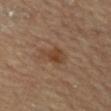{
  "biopsy_status": "not biopsied; imaged during a skin examination",
  "lighting": "cross-polarized",
  "lesion_size": {
    "long_diameter_mm_approx": 4.0
  },
  "site": "mid back",
  "patient": {
    "sex": "male",
    "age_approx": "85+"
  },
  "image": {
    "source": "total-body photography crop",
    "field_of_view_mm": 15
  }
}